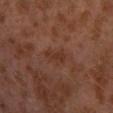Q: Is there a histopathology result?
A: total-body-photography surveillance lesion; no biopsy
Q: Lesion location?
A: the left lower leg
Q: What are the patient's age and sex?
A: male, about 30 years old
Q: How large is the lesion?
A: ~3 mm (longest diameter)
Q: What kind of image is this?
A: 15 mm crop, total-body photography
Q: How was the tile lit?
A: cross-polarized illumination